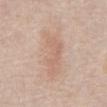<case>
<biopsy_status>not biopsied; imaged during a skin examination</biopsy_status>
<image>
  <source>total-body photography crop</source>
  <field_of_view_mm>15</field_of_view_mm>
</image>
<lighting>white-light</lighting>
<patient>
  <sex>male</sex>
  <age_approx>75</age_approx>
</patient>
<lesion_size>
  <long_diameter_mm_approx>7.0</long_diameter_mm_approx>
</lesion_size>
<site>abdomen</site>
<automated_metrics>
  <eccentricity>0.9</eccentricity>
  <shape_asymmetry>0.25</shape_asymmetry>
</automated_metrics>
</case>